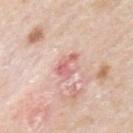Case summary:
* notes: imaged on a skin check; not biopsied
* site: the left upper arm
* patient: male, aged around 75
* illumination: white-light illumination
* size: ≈3 mm
* imaging modality: ~15 mm crop, total-body skin-cancer survey
* TBP lesion metrics: radial color variation of about 1